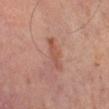follow-up = imaged on a skin check; not biopsied
lesion diameter = ≈4 mm
location = the left thigh
patient = male, about 65 years old
automated lesion analysis = a footprint of about 5 mm², a shape eccentricity near 0.95, and two-axis asymmetry of about 0.25
acquisition = total-body-photography crop, ~15 mm field of view
tile lighting = cross-polarized